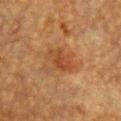This lesion was catalogued during total-body skin photography and was not selected for biopsy. Cropped from a total-body skin-imaging series; the visible field is about 15 mm. Automated image analysis of the tile measured an eccentricity of roughly 0.8 and a shape-asymmetry score of about 0.25 (0 = symmetric). On the front of the torso. A female patient, about 55 years old. The recorded lesion diameter is about 3.5 mm.